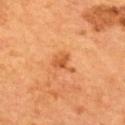Background: About 3 mm across. The lesion is located on the mid back. Imaged with cross-polarized lighting. Cropped from a total-body skin-imaging series; the visible field is about 15 mm. A male subject, about 55 years old. The total-body-photography lesion software estimated border irregularity of about 5 on a 0–10 scale, a within-lesion color-variation index near 2.5/10, and a peripheral color-asymmetry measure near 1. It also reported a nevus-likeness score of about 50/100.